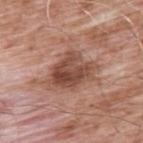Q: Was this lesion biopsied?
A: total-body-photography surveillance lesion; no biopsy
Q: What is the lesion's diameter?
A: about 5.5 mm
Q: What is the anatomic site?
A: the upper back
Q: How was this image acquired?
A: total-body-photography crop, ~15 mm field of view
Q: Who is the patient?
A: male, approximately 60 years of age